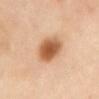Q: Was a biopsy performed?
A: imaged on a skin check; not biopsied
Q: Lesion location?
A: the abdomen
Q: What is the imaging modality?
A: 15 mm crop, total-body photography
Q: What lighting was used for the tile?
A: cross-polarized illumination
Q: Who is the patient?
A: female, about 50 years old